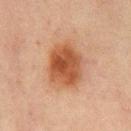Findings:
* biopsy status · catalogued during a skin exam; not biopsied
* lighting · cross-polarized illumination
* anatomic site · the mid back
* imaging modality · ~15 mm crop, total-body skin-cancer survey
* patient · male, about 65 years old
* lesion size · ≈5 mm